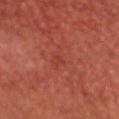<case>
  <site>head or neck</site>
  <automated_metrics>
    <cielab_L>38</cielab_L>
    <cielab_a>33</cielab_a>
    <cielab_b>32</cielab_b>
    <vs_skin_darker_L>4.0</vs_skin_darker_L>
    <vs_skin_contrast_norm>4.0</vs_skin_contrast_norm>
    <border_irregularity_0_10>2.5</border_irregularity_0_10>
    <color_variation_0_10>0.0</color_variation_0_10>
    <peripheral_color_asymmetry>0.0</peripheral_color_asymmetry>
    <nevus_likeness_0_100>0</nevus_likeness_0_100>
  </automated_metrics>
  <lesion_size>
    <long_diameter_mm_approx>1.0</long_diameter_mm_approx>
  </lesion_size>
  <lighting>cross-polarized</lighting>
  <image>
    <source>total-body photography crop</source>
    <field_of_view_mm>15</field_of_view_mm>
  </image>
  <patient>
    <sex>male</sex>
    <age_approx>60</age_approx>
  </patient>
</case>